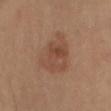Case summary:
– biopsy status: imaged on a skin check; not biopsied
– automated metrics: a lesion area of about 19 mm² and an outline eccentricity of about 0.65 (0 = round, 1 = elongated); a lesion color around L≈48 a*≈20 b*≈29 in CIELAB, a lesion–skin lightness drop of about 7, and a lesion-to-skin contrast of about 5.5 (normalized; higher = more distinct); an automated nevus-likeness rating near 55 out of 100 and lesion-presence confidence of about 100/100
– patient: female, about 70 years old
– site: the leg
– image: ~15 mm crop, total-body skin-cancer survey
– illumination: cross-polarized
– size: ≈6 mm